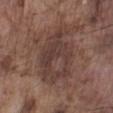Imaged during a routine full-body skin examination; the lesion was not biopsied and no histopathology is available.
A region of skin cropped from a whole-body photographic capture, roughly 15 mm wide.
Approximately 7.5 mm at its widest.
The lesion is on the left lower leg.
A male patient aged 73 to 77.